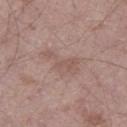The lesion was tiled from a total-body skin photograph and was not biopsied. Located on the left thigh. Captured under white-light illumination. Cropped from a total-body skin-imaging series; the visible field is about 15 mm. The recorded lesion diameter is about 4.5 mm. Automated image analysis of the tile measured an area of roughly 7.5 mm², an eccentricity of roughly 0.9, and a symmetry-axis asymmetry near 0.55. It also reported a lesion color around L≈54 a*≈18 b*≈22 in CIELAB, roughly 6 lightness units darker than nearby skin, and a normalized lesion–skin contrast near 4.5. The software also gave a border-irregularity rating of about 7.5/10, internal color variation of about 1.5 on a 0–10 scale, and peripheral color asymmetry of about 0.5. A male subject, aged around 50.